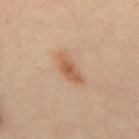This lesion was catalogued during total-body skin photography and was not selected for biopsy.
The lesion-visualizer software estimated a lesion area of about 6 mm² and an eccentricity of roughly 0.9. And it measured an average lesion color of about L≈57 a*≈20 b*≈33 (CIELAB), roughly 9 lightness units darker than nearby skin, and a lesion-to-skin contrast of about 7 (normalized; higher = more distinct). The software also gave a detector confidence of about 100 out of 100 that the crop contains a lesion.
Located on the mid back.
A 15 mm crop from a total-body photograph taken for skin-cancer surveillance.
A male subject, aged around 50.
Imaged with cross-polarized lighting.
About 4.5 mm across.